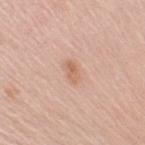• workup: no biopsy performed (imaged during a skin exam)
• size: about 3 mm
• anatomic site: the right upper arm
• tile lighting: white-light
• image-analysis metrics: a lesion area of about 3.5 mm² and a shape eccentricity near 0.85; a lesion color around L≈63 a*≈21 b*≈31 in CIELAB and a lesion–skin lightness drop of about 8; a border-irregularity index near 2.5/10, a within-lesion color-variation index near 3.5/10, and radial color variation of about 1.5; a classifier nevus-likeness of about 15/100 and lesion-presence confidence of about 100/100
• subject: female, aged 63 to 67
• acquisition: 15 mm crop, total-body photography